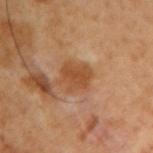No biopsy was performed on this lesion — it was imaged during a full skin examination and was not determined to be concerning. A roughly 15 mm field-of-view crop from a total-body skin photograph. On the right upper arm. The patient is a male aged 48 to 52. Measured at roughly 3.5 mm in maximum diameter.